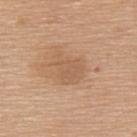<record>
  <biopsy_status>not biopsied; imaged during a skin examination</biopsy_status>
  <site>upper back</site>
  <patient>
    <sex>female</sex>
    <age_approx>60</age_approx>
  </patient>
  <image>
    <source>total-body photography crop</source>
    <field_of_view_mm>15</field_of_view_mm>
  </image>
</record>